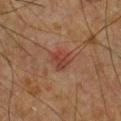Notes:
* patient · male, aged approximately 50
* lighting · cross-polarized
* anatomic site · the front of the torso
* acquisition · ~15 mm tile from a whole-body skin photo
* size · about 2.5 mm